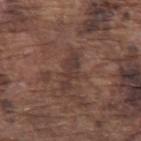Notes:
* tile lighting · white-light illumination
* location · the arm
* automated metrics · a footprint of about 6 mm² and a shape-asymmetry score of about 0.3 (0 = symmetric); a mean CIELAB color near L≈36 a*≈17 b*≈21 and about 7 CIELAB-L* units darker than the surrounding skin; a border-irregularity index near 5.5/10 and a within-lesion color-variation index near 2/10
* image source · total-body-photography crop, ~15 mm field of view
* patient · male, about 75 years old
* lesion diameter · about 4.5 mm